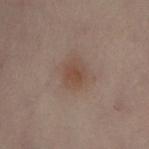biopsy status = no biopsy performed (imaged during a skin exam); illumination = cross-polarized illumination; location = the left lower leg; patient = female, aged 48–52; lesion diameter = about 3 mm; acquisition = 15 mm crop, total-body photography.